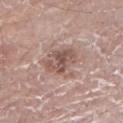follow-up=catalogued during a skin exam; not biopsied | illumination=white-light illumination | lesion size=≈4 mm | patient=male, aged 78 to 82 | image source=~15 mm tile from a whole-body skin photo | location=the leg.